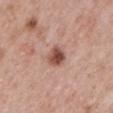Clinical impression:
Recorded during total-body skin imaging; not selected for excision or biopsy.
Acquisition and patient details:
An algorithmic analysis of the crop reported a lesion area of about 5 mm², an eccentricity of roughly 0.45, and two-axis asymmetry of about 0.25. It also reported an automated nevus-likeness rating near 95 out of 100 and lesion-presence confidence of about 100/100. This image is a 15 mm lesion crop taken from a total-body photograph. On the mid back. Approximately 2.5 mm at its widest. A male patient aged approximately 65.